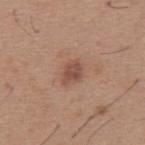  biopsy_status: not biopsied; imaged during a skin examination
  patient:
    sex: male
    age_approx: 65
  automated_metrics:
    area_mm2_approx: 4.5
    eccentricity: 0.7
    shape_asymmetry: 0.3
    border_irregularity_0_10: 2.5
    color_variation_0_10: 2.5
    peripheral_color_asymmetry: 1.0
    lesion_detection_confidence_0_100: 100
  image:
    source: total-body photography crop
    field_of_view_mm: 15
  site: upper back
  lesion_size:
    long_diameter_mm_approx: 3.0